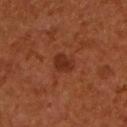| field | value |
|---|---|
| workup | catalogued during a skin exam; not biopsied |
| acquisition | 15 mm crop, total-body photography |
| anatomic site | the upper back |
| patient | male, aged 53–57 |